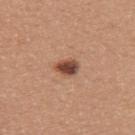Background:
From the upper back. The tile uses white-light illumination. A lesion tile, about 15 mm wide, cut from a 3D total-body photograph. A female patient, aged around 55. The recorded lesion diameter is about 3 mm.